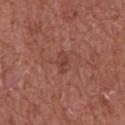Recorded during total-body skin imaging; not selected for excision or biopsy. On the abdomen. A 15 mm close-up extracted from a 3D total-body photography capture. The subject is a male aged 63–67.